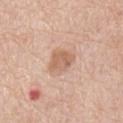follow-up: imaged on a skin check; not biopsied | size: ≈3 mm | imaging modality: ~15 mm tile from a whole-body skin photo | anatomic site: the abdomen | illumination: white-light illumination | TBP lesion metrics: a lesion area of about 6 mm²; a within-lesion color-variation index near 3/10 and a peripheral color-asymmetry measure near 1 | subject: female, aged 73 to 77.